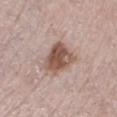Q: Lesion location?
A: the leg
Q: How was this image acquired?
A: 15 mm crop, total-body photography
Q: What lighting was used for the tile?
A: white-light illumination
Q: Patient demographics?
A: female, aged around 65
Q: Lesion size?
A: ≈4 mm